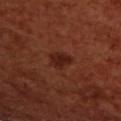The lesion was photographed on a routine skin check and not biopsied; there is no pathology result.
Longest diameter approximately 3 mm.
A 15 mm close-up extracted from a 3D total-body photography capture.
A female subject in their mid- to late 50s.
The tile uses cross-polarized illumination.
The lesion is located on the upper back.
The total-body-photography lesion software estimated an area of roughly 4.5 mm², a shape eccentricity near 0.7, and a shape-asymmetry score of about 0.25 (0 = symmetric). It also reported a lesion color around L≈24 a*≈25 b*≈27 in CIELAB, a lesion–skin lightness drop of about 8, and a normalized lesion–skin contrast near 8.5. The analysis additionally found a border-irregularity rating of about 2.5/10, a within-lesion color-variation index near 2/10, and a peripheral color-asymmetry measure near 1. And it measured a detector confidence of about 100 out of 100 that the crop contains a lesion.